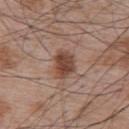– workup — catalogued during a skin exam; not biopsied
– size — about 3.5 mm
– acquisition — ~15 mm crop, total-body skin-cancer survey
– subject — male, about 65 years old
– TBP lesion metrics — a border-irregularity index near 2.5/10, internal color variation of about 3.5 on a 0–10 scale, and radial color variation of about 1
– illumination — white-light illumination
– body site — the upper back